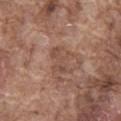Assessment: Captured during whole-body skin photography for melanoma surveillance; the lesion was not biopsied. Image and clinical context: The lesion is located on the abdomen. A region of skin cropped from a whole-body photographic capture, roughly 15 mm wide. A male patient aged approximately 75.